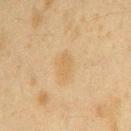About 2.5 mm across.
The lesion-visualizer software estimated an area of roughly 3.5 mm², an outline eccentricity of about 0.8 (0 = round, 1 = elongated), and a shape-asymmetry score of about 0.35 (0 = symmetric). It also reported a lesion color around L≈53 a*≈13 b*≈35 in CIELAB, about 5 CIELAB-L* units darker than the surrounding skin, and a normalized lesion–skin contrast near 5. The analysis additionally found a border-irregularity rating of about 3/10, a within-lesion color-variation index near 1/10, and a peripheral color-asymmetry measure near 0.5. It also reported a nevus-likeness score of about 5/100.
A 15 mm crop from a total-body photograph taken for skin-cancer surveillance.
The tile uses cross-polarized illumination.
The lesion is on the right upper arm.
The subject is a female about 40 years old.